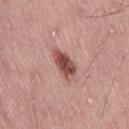Imaged during a routine full-body skin examination; the lesion was not biopsied and no histopathology is available.
This image is a 15 mm lesion crop taken from a total-body photograph.
The patient is a male approximately 45 years of age.
On the leg.